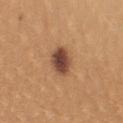image-analysis metrics: an area of roughly 7.5 mm², an eccentricity of roughly 0.4, and a shape-asymmetry score of about 0.15 (0 = symmetric); a mean CIELAB color near L≈46 a*≈21 b*≈30, a lesion–skin lightness drop of about 15, and a normalized border contrast of about 12; a border-irregularity rating of about 1.5/10 and a peripheral color-asymmetry measure near 1.5; a classifier nevus-likeness of about 80/100 and lesion-presence confidence of about 100/100
anatomic site: the arm
image source: ~15 mm crop, total-body skin-cancer survey
diameter: about 3 mm
patient: female, in their mid- to late 20s